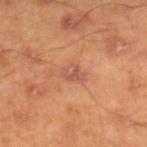subject: male, aged 43–47 | site: the leg | size: about 2.5 mm | tile lighting: cross-polarized | image: 15 mm crop, total-body photography | automated metrics: a footprint of about 3.5 mm², an outline eccentricity of about 0.7 (0 = round, 1 = elongated), and two-axis asymmetry of about 0.25; a lesion color around L≈54 a*≈26 b*≈31 in CIELAB; internal color variation of about 2.5 on a 0–10 scale and a peripheral color-asymmetry measure near 1; a nevus-likeness score of about 0/100 and lesion-presence confidence of about 100/100.